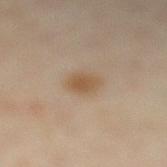Notes:
– biopsy status — total-body-photography surveillance lesion; no biopsy
– location — the leg
– lesion size — about 2.5 mm
– lighting — cross-polarized illumination
– image — ~15 mm tile from a whole-body skin photo
– patient — female, aged approximately 35